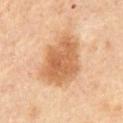– biopsy status: catalogued during a skin exam; not biopsied
– patient: male, about 65 years old
– anatomic site: the abdomen
– size: ~6.5 mm (longest diameter)
– image: ~15 mm tile from a whole-body skin photo
– illumination: cross-polarized